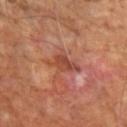Assessment:
The lesion was photographed on a routine skin check and not biopsied; there is no pathology result.
Context:
Cropped from a whole-body photographic skin survey; the tile spans about 15 mm. The patient is a male in their mid- to late 60s. From the left upper arm.